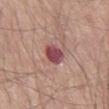Captured during whole-body skin photography for melanoma surveillance; the lesion was not biopsied. A lesion tile, about 15 mm wide, cut from a 3D total-body photograph. The lesion's longest dimension is about 3.5 mm. The tile uses white-light illumination. The lesion is located on the right thigh. The patient is a male in their 80s.